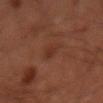The lesion was photographed on a routine skin check and not biopsied; there is no pathology result. Located on the arm. Captured under cross-polarized illumination. A male subject, aged around 70. Automated tile analysis of the lesion measured a lesion color around L≈24 a*≈18 b*≈22 in CIELAB and a normalized lesion–skin contrast near 4.5. A close-up tile cropped from a whole-body skin photograph, about 15 mm across.